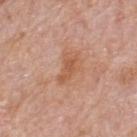Q: What are the patient's age and sex?
A: male, in their mid- to late 70s
Q: Lesion location?
A: the back
Q: How was this image acquired?
A: total-body-photography crop, ~15 mm field of view